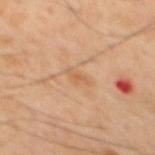The lesion was tiled from a total-body skin photograph and was not biopsied. The lesion is on the mid back. Longest diameter approximately 2.5 mm. A male subject, in their mid-40s. This is a cross-polarized tile. Automated image analysis of the tile measured an automated nevus-likeness rating near 0 out of 100 and lesion-presence confidence of about 100/100. A roughly 15 mm field-of-view crop from a total-body skin photograph.